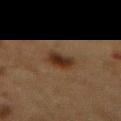The lesion was photographed on a routine skin check and not biopsied; there is no pathology result.
The recorded lesion diameter is about 2.5 mm.
The lesion is on the abdomen.
A female subject, in their 50s.
This image is a 15 mm lesion crop taken from a total-body photograph.
This is a cross-polarized tile.
An algorithmic analysis of the crop reported a border-irregularity index near 2/10, a within-lesion color-variation index near 4/10, and peripheral color asymmetry of about 1.5. The analysis additionally found an automated nevus-likeness rating near 95 out of 100 and lesion-presence confidence of about 100/100.